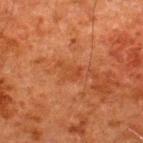workup = imaged on a skin check; not biopsied | illumination = cross-polarized | anatomic site = the upper back | image = ~15 mm crop, total-body skin-cancer survey | subject = male, aged around 60 | automated metrics = about 5 CIELAB-L* units darker than the surrounding skin and a normalized lesion–skin contrast near 5.